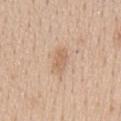{
  "biopsy_status": "not biopsied; imaged during a skin examination",
  "image": {
    "source": "total-body photography crop",
    "field_of_view_mm": 15
  },
  "site": "mid back",
  "patient": {
    "sex": "male",
    "age_approx": 60
  },
  "lighting": "white-light",
  "lesion_size": {
    "long_diameter_mm_approx": 3.5
  }
}